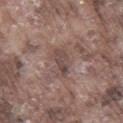{
  "biopsy_status": "not biopsied; imaged during a skin examination",
  "lighting": "white-light",
  "automated_metrics": {
    "area_mm2_approx": 4.0,
    "eccentricity": 0.95,
    "shape_asymmetry": 0.4,
    "vs_skin_darker_L": 8.0,
    "color_variation_0_10": 0.5,
    "peripheral_color_asymmetry": 0.0
  },
  "patient": {
    "sex": "male",
    "age_approx": 75
  },
  "site": "leg",
  "lesion_size": {
    "long_diameter_mm_approx": 3.5
  },
  "image": {
    "source": "total-body photography crop",
    "field_of_view_mm": 15
  }
}